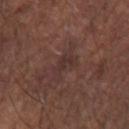biopsy status: total-body-photography surveillance lesion; no biopsy | tile lighting: white-light | lesion diameter: about 4 mm | anatomic site: the right forearm | patient: male, about 65 years old | automated lesion analysis: a footprint of about 6 mm² and a shape-asymmetry score of about 0.45 (0 = symmetric); a mean CIELAB color near L≈32 a*≈17 b*≈19; a nevus-likeness score of about 0/100 and a detector confidence of about 90 out of 100 that the crop contains a lesion | imaging modality: total-body-photography crop, ~15 mm field of view.